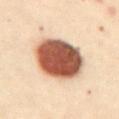workup=catalogued during a skin exam; not biopsied | image=~15 mm tile from a whole-body skin photo | patient=female, about 30 years old | body site=the abdomen.